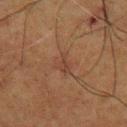Assessment:
The lesion was tiled from a total-body skin photograph and was not biopsied.
Clinical summary:
Captured under cross-polarized illumination. The recorded lesion diameter is about 2.5 mm. The lesion is on the upper back. The lesion-visualizer software estimated a footprint of about 4 mm². A male patient aged approximately 60. Cropped from a whole-body photographic skin survey; the tile spans about 15 mm.